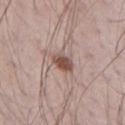  biopsy_status: not biopsied; imaged during a skin examination